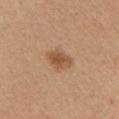Q: Is there a histopathology result?
A: no biopsy performed (imaged during a skin exam)
Q: What kind of image is this?
A: ~15 mm tile from a whole-body skin photo
Q: Lesion location?
A: the left upper arm
Q: Patient demographics?
A: female, in their mid- to late 40s
Q: What is the lesion's diameter?
A: ~3 mm (longest diameter)
Q: What did automated image analysis measure?
A: a footprint of about 5.5 mm², an outline eccentricity of about 0.7 (0 = round, 1 = elongated), and a symmetry-axis asymmetry near 0.2
Q: Illumination type?
A: white-light